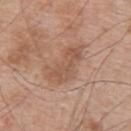workup: total-body-photography surveillance lesion; no biopsy | site: the upper back | diameter: ≈6 mm | patient: male, aged 63–67 | image source: total-body-photography crop, ~15 mm field of view.